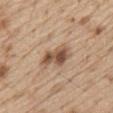workup: imaged on a skin check; not biopsied | location: the abdomen | patient: male, aged 68–72 | image: ~15 mm tile from a whole-body skin photo.